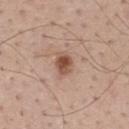This lesion was catalogued during total-body skin photography and was not selected for biopsy. The recorded lesion diameter is about 3 mm. Imaged with white-light lighting. An algorithmic analysis of the crop reported a footprint of about 4.5 mm² and an eccentricity of roughly 0.65. It also reported a mean CIELAB color near L≈51 a*≈21 b*≈29 and a lesion–skin lightness drop of about 13. A male patient approximately 45 years of age. Cropped from a total-body skin-imaging series; the visible field is about 15 mm. The lesion is on the upper back.